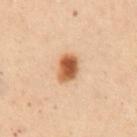biopsy_status: not biopsied; imaged during a skin examination
lesion_size:
  long_diameter_mm_approx: 3.5
automated_metrics:
  cielab_L: 49
  cielab_a: 21
  cielab_b: 33
image:
  source: total-body photography crop
  field_of_view_mm: 15
patient:
  sex: female
  age_approx: 50
site: mid back